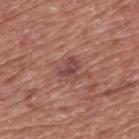| key | value |
|---|---|
| notes | catalogued during a skin exam; not biopsied |
| diameter | ~3 mm (longest diameter) |
| subject | male, aged around 75 |
| location | the upper back |
| acquisition | 15 mm crop, total-body photography |
| illumination | white-light illumination |